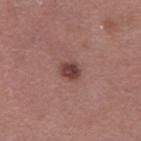Clinical impression:
Part of a total-body skin-imaging series; this lesion was reviewed on a skin check and was not flagged for biopsy.
Clinical summary:
On the left thigh. Cropped from a whole-body photographic skin survey; the tile spans about 15 mm. A female subject, aged 38 to 42.